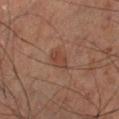biopsy_status: not biopsied; imaged during a skin examination
automated_metrics:
  cielab_L: 31
  cielab_a: 17
  cielab_b: 22
  vs_skin_darker_L: 6.0
  vs_skin_contrast_norm: 6.0
lighting: cross-polarized
patient:
  sex: male
  age_approx: 60
site: leg
lesion_size:
  long_diameter_mm_approx: 3.0
image:
  source: total-body photography crop
  field_of_view_mm: 15